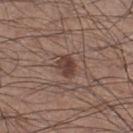This lesion was catalogued during total-body skin photography and was not selected for biopsy.
On the right lower leg.
The tile uses white-light illumination.
A male patient, aged 33 to 37.
The total-body-photography lesion software estimated an eccentricity of roughly 0.6 and two-axis asymmetry of about 0.25. The analysis additionally found a lesion color around L≈40 a*≈18 b*≈23 in CIELAB, about 10 CIELAB-L* units darker than the surrounding skin, and a normalized lesion–skin contrast near 8.5. The software also gave an automated nevus-likeness rating near 70 out of 100.
The recorded lesion diameter is about 2.5 mm.
A 15 mm close-up tile from a total-body photography series done for melanoma screening.